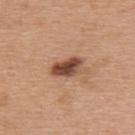Case summary:
– follow-up — no biopsy performed (imaged during a skin exam)
– subject — female, aged around 40
– lighting — white-light illumination
– imaging modality — ~15 mm tile from a whole-body skin photo
– anatomic site — the back